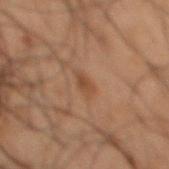This lesion was catalogued during total-body skin photography and was not selected for biopsy. A lesion tile, about 15 mm wide, cut from a 3D total-body photograph. Approximately 2.5 mm at its widest. This is a cross-polarized tile. A male subject approximately 50 years of age. The lesion is located on the back. The total-body-photography lesion software estimated a mean CIELAB color near L≈35 a*≈16 b*≈26 and roughly 6 lightness units darker than nearby skin. The software also gave a border-irregularity index near 4/10, a within-lesion color-variation index near 0.5/10, and peripheral color asymmetry of about 0.